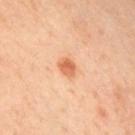Assessment: No biopsy was performed on this lesion — it was imaged during a full skin examination and was not determined to be concerning. Background: Cropped from a total-body skin-imaging series; the visible field is about 15 mm. The tile uses cross-polarized illumination. A male subject, in their 40s. The lesion is on the front of the torso. The total-body-photography lesion software estimated a lesion color around L≈52 a*≈23 b*≈32 in CIELAB. It also reported a classifier nevus-likeness of about 95/100 and a detector confidence of about 100 out of 100 that the crop contains a lesion. Measured at roughly 2.5 mm in maximum diameter.